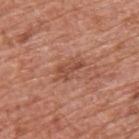notes = total-body-photography surveillance lesion; no biopsy | imaging modality = ~15 mm crop, total-body skin-cancer survey | anatomic site = the upper back | patient = male, aged approximately 65 | size = about 3.5 mm.